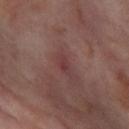Clinical impression: Imaged during a routine full-body skin examination; the lesion was not biopsied and no histopathology is available. Clinical summary: The lesion is on the right thigh. A 15 mm close-up extracted from a 3D total-body photography capture. A female patient, aged approximately 55. Approximately 2.5 mm at its widest.